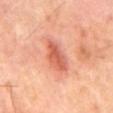Cropped from a whole-body photographic skin survey; the tile spans about 15 mm.
On the mid back.
A male subject, approximately 65 years of age.